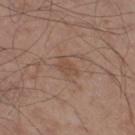biopsy_status: not biopsied; imaged during a skin examination
patient:
  sex: male
  age_approx: 55
lighting: white-light
site: leg
image:
  source: total-body photography crop
  field_of_view_mm: 15